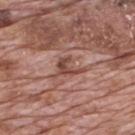The lesion was tiled from a total-body skin photograph and was not biopsied. The patient is a male aged approximately 70. A lesion tile, about 15 mm wide, cut from a 3D total-body photograph. On the mid back.